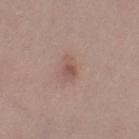Impression: Part of a total-body skin-imaging series; this lesion was reviewed on a skin check and was not flagged for biopsy. Background: On the left thigh. A 15 mm close-up tile from a total-body photography series done for melanoma screening. A female patient, aged around 35.